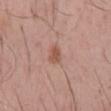Impression: Captured during whole-body skin photography for melanoma surveillance; the lesion was not biopsied. Acquisition and patient details: The subject is a male in their mid-50s. A roughly 15 mm field-of-view crop from a total-body skin photograph. On the mid back. The lesion-visualizer software estimated an area of roughly 4 mm², an eccentricity of roughly 0.65, and a shape-asymmetry score of about 0.25 (0 = symmetric). The software also gave a mean CIELAB color near L≈54 a*≈22 b*≈29 and a lesion-to-skin contrast of about 7 (normalized; higher = more distinct). The analysis additionally found an automated nevus-likeness rating near 40 out of 100 and lesion-presence confidence of about 100/100.